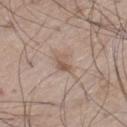No biopsy was performed on this lesion — it was imaged during a full skin examination and was not determined to be concerning. A male subject aged approximately 70. The tile uses white-light illumination. A region of skin cropped from a whole-body photographic capture, roughly 15 mm wide. The recorded lesion diameter is about 2.5 mm. Located on the leg.